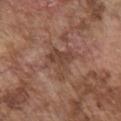| feature | finding |
|---|---|
| workup | no biopsy performed (imaged during a skin exam) |
| lesion size | about 4 mm |
| TBP lesion metrics | a lesion area of about 9 mm² and a symmetry-axis asymmetry near 0.55; a classifier nevus-likeness of about 0/100 and lesion-presence confidence of about 95/100 |
| subject | male, aged approximately 75 |
| location | the chest |
| tile lighting | white-light |
| acquisition | ~15 mm crop, total-body skin-cancer survey |